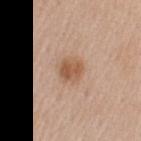follow-up = total-body-photography surveillance lesion; no biopsy | patient = female, in their mid- to late 40s | site = the arm | automated metrics = an automated nevus-likeness rating near 90 out of 100 and a detector confidence of about 100 out of 100 that the crop contains a lesion | acquisition = ~15 mm tile from a whole-body skin photo | tile lighting = white-light | size = about 2.5 mm.